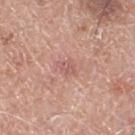notes=total-body-photography surveillance lesion; no biopsy | anatomic site=the right thigh | lesion size=about 2.5 mm | patient=male, aged 78 to 82 | image=~15 mm crop, total-body skin-cancer survey.